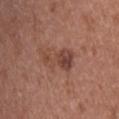Notes:
– biopsy status — no biopsy performed (imaged during a skin exam)
– patient — female, about 40 years old
– site — the upper back
– acquisition — 15 mm crop, total-body photography